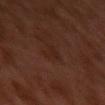Recorded during total-body skin imaging; not selected for excision or biopsy. A male subject, about 30 years old. Cropped from a total-body skin-imaging series; the visible field is about 15 mm. On the left upper arm.